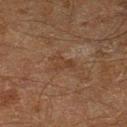Recorded during total-body skin imaging; not selected for excision or biopsy. Located on the left lower leg. Automated tile analysis of the lesion measured a mean CIELAB color near L≈29 a*≈15 b*≈24, a lesion–skin lightness drop of about 5, and a lesion-to-skin contrast of about 5 (normalized; higher = more distinct). The recorded lesion diameter is about 2.5 mm. A male subject aged 58–62. This image is a 15 mm lesion crop taken from a total-body photograph. The tile uses cross-polarized illumination.